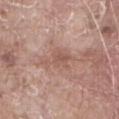notes: imaged on a skin check; not biopsied | location: the abdomen | acquisition: 15 mm crop, total-body photography | patient: male, aged around 80 | lighting: white-light illumination.